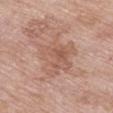Captured during whole-body skin photography for melanoma surveillance; the lesion was not biopsied. Located on the chest. About 5.5 mm across. A female patient aged approximately 70. The total-body-photography lesion software estimated an average lesion color of about L≈57 a*≈20 b*≈28 (CIELAB) and about 7 CIELAB-L* units darker than the surrounding skin. It also reported a border-irregularity rating of about 5.5/10, internal color variation of about 4 on a 0–10 scale, and peripheral color asymmetry of about 1.5. This is a white-light tile. A region of skin cropped from a whole-body photographic capture, roughly 15 mm wide.